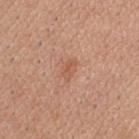| field | value |
|---|---|
| workup | imaged on a skin check; not biopsied |
| patient | male, roughly 40 years of age |
| location | the head or neck |
| image | ~15 mm tile from a whole-body skin photo |
| lighting | white-light |